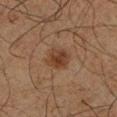Impression:
No biopsy was performed on this lesion — it was imaged during a full skin examination and was not determined to be concerning.
Clinical summary:
Automated image analysis of the tile measured an outline eccentricity of about 0.45 (0 = round, 1 = elongated) and a shape-asymmetry score of about 0.2 (0 = symmetric). The software also gave an automated nevus-likeness rating near 80 out of 100. The subject is a male aged around 65. On the left lower leg. This is a cross-polarized tile. A 15 mm crop from a total-body photograph taken for skin-cancer surveillance. Measured at roughly 3 mm in maximum diameter.